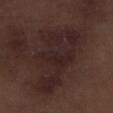Clinical impression: Captured during whole-body skin photography for melanoma surveillance; the lesion was not biopsied. Context: Approximately 10.5 mm at its widest. The subject is a male roughly 70 years of age. The lesion is on the leg. A 15 mm close-up extracted from a 3D total-body photography capture.